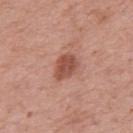| key | value |
|---|---|
| workup | total-body-photography surveillance lesion; no biopsy |
| lesion size | about 3.5 mm |
| body site | the right upper arm |
| patient | male, in their 60s |
| imaging modality | 15 mm crop, total-body photography |